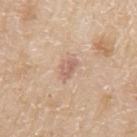Imaged during a routine full-body skin examination; the lesion was not biopsied and no histopathology is available.